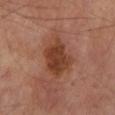Imaged during a routine full-body skin examination; the lesion was not biopsied and no histopathology is available.
Captured under cross-polarized illumination.
From the left lower leg.
About 6.5 mm across.
An algorithmic analysis of the crop reported a shape eccentricity near 0.8 and a shape-asymmetry score of about 0.3 (0 = symmetric). The software also gave a mean CIELAB color near L≈40 a*≈23 b*≈29, roughly 10 lightness units darker than nearby skin, and a lesion-to-skin contrast of about 9 (normalized; higher = more distinct). The software also gave a within-lesion color-variation index near 4.5/10 and a peripheral color-asymmetry measure near 1.5. The analysis additionally found a nevus-likeness score of about 45/100 and a lesion-detection confidence of about 100/100.
A male patient aged approximately 70.
This image is a 15 mm lesion crop taken from a total-body photograph.